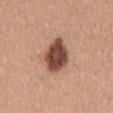Findings:
– notes — no biopsy performed (imaged during a skin exam)
– site — the mid back
– lesion size — about 5 mm
– TBP lesion metrics — a border-irregularity rating of about 2/10, a within-lesion color-variation index near 4.5/10, and a peripheral color-asymmetry measure near 1.5; a classifier nevus-likeness of about 70/100 and a detector confidence of about 100 out of 100 that the crop contains a lesion
– patient — female, aged approximately 30
– image source — 15 mm crop, total-body photography
– lighting — white-light